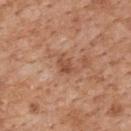Clinical impression:
No biopsy was performed on this lesion — it was imaged during a full skin examination and was not determined to be concerning.
Clinical summary:
A 15 mm close-up tile from a total-body photography series done for melanoma screening. The recorded lesion diameter is about 2.5 mm. A male patient, aged approximately 60. The lesion is on the upper back.